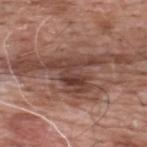Notes:
– follow-up — no biopsy performed (imaged during a skin exam)
– location — the upper back
– lesion size — ≈6 mm
– TBP lesion metrics — a footprint of about 13 mm² and two-axis asymmetry of about 0.55; a normalized border contrast of about 9.5; a border-irregularity index near 8/10 and a peripheral color-asymmetry measure near 2; a nevus-likeness score of about 15/100 and a lesion-detection confidence of about 95/100
– illumination — white-light illumination
– imaging modality — 15 mm crop, total-body photography
– patient — male, aged around 60